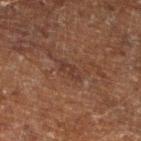Clinical impression: The lesion was tiled from a total-body skin photograph and was not biopsied. Acquisition and patient details: A male patient about 60 years old. The lesion's longest dimension is about 3 mm. Cropped from a total-body skin-imaging series; the visible field is about 15 mm. The lesion is on the left lower leg.